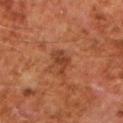This lesion was catalogued during total-body skin photography and was not selected for biopsy. A male patient, approximately 60 years of age. The recorded lesion diameter is about 3.5 mm. From the arm. This image is a 15 mm lesion crop taken from a total-body photograph.